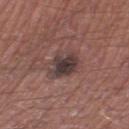Impression:
This lesion was catalogued during total-body skin photography and was not selected for biopsy.
Background:
The tile uses white-light illumination. The recorded lesion diameter is about 3.5 mm. Located on the right thigh. A close-up tile cropped from a whole-body skin photograph, about 15 mm across. A male subject in their mid-50s. The total-body-photography lesion software estimated an average lesion color of about L≈37 a*≈15 b*≈16 (CIELAB), about 11 CIELAB-L* units darker than the surrounding skin, and a normalized lesion–skin contrast near 10.5. And it measured a nevus-likeness score of about 20/100 and a lesion-detection confidence of about 100/100.